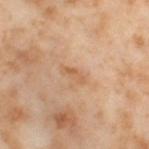Imaged during a routine full-body skin examination; the lesion was not biopsied and no histopathology is available.
A 15 mm close-up tile from a total-body photography series done for melanoma screening.
The subject is a female in their mid- to late 50s.
The lesion's longest dimension is about 3 mm.
The lesion is located on the leg.
The tile uses cross-polarized illumination.